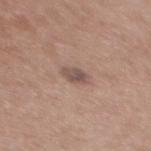Clinical impression:
The lesion was photographed on a routine skin check and not biopsied; there is no pathology result.
Background:
A 15 mm close-up extracted from a 3D total-body photography capture. Located on the upper back. The patient is a female approximately 40 years of age. The recorded lesion diameter is about 2.5 mm.